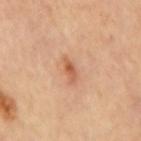Findings:
• workup — total-body-photography surveillance lesion; no biopsy
• location — the mid back
• acquisition — 15 mm crop, total-body photography